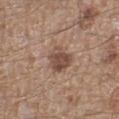This lesion was catalogued during total-body skin photography and was not selected for biopsy.
Cropped from a whole-body photographic skin survey; the tile spans about 15 mm.
The subject is a male aged 63 to 67.
The lesion is on the left lower leg.
This is a white-light tile.
Automated tile analysis of the lesion measured a footprint of about 7 mm². The analysis additionally found a border-irregularity index near 3/10 and radial color variation of about 1.5. It also reported an automated nevus-likeness rating near 70 out of 100 and a detector confidence of about 100 out of 100 that the crop contains a lesion.
The recorded lesion diameter is about 3 mm.